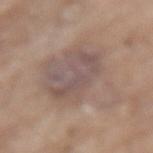<lesion>
  <biopsy_status>not biopsied; imaged during a skin examination</biopsy_status>
  <patient>
    <sex>male</sex>
    <age_approx>80</age_approx>
  </patient>
  <lesion_size>
    <long_diameter_mm_approx>9.0</long_diameter_mm_approx>
  </lesion_size>
  <lighting>white-light</lighting>
  <automated_metrics>
    <area_mm2_approx>35.0</area_mm2_approx>
    <shape_asymmetry>0.4</shape_asymmetry>
    <border_irregularity_0_10>7.0</border_irregularity_0_10>
    <color_variation_0_10>4.5</color_variation_0_10>
    <peripheral_color_asymmetry>1.5</peripheral_color_asymmetry>
  </automated_metrics>
  <site>lower back</site>
  <image>
    <source>total-body photography crop</source>
    <field_of_view_mm>15</field_of_view_mm>
  </image>
</lesion>